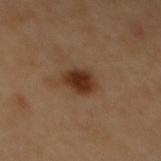lighting: cross-polarized
site: upper back
lesion_size:
  long_diameter_mm_approx: 3.5
image:
  source: total-body photography crop
  field_of_view_mm: 15
patient:
  sex: female
  age_approx: 60